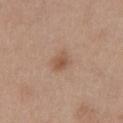{
  "biopsy_status": "not biopsied; imaged during a skin examination",
  "image": {
    "source": "total-body photography crop",
    "field_of_view_mm": 15
  },
  "lesion_size": {
    "long_diameter_mm_approx": 2.5
  },
  "patient": {
    "sex": "female",
    "age_approx": 40
  },
  "automated_metrics": {
    "area_mm2_approx": 4.5,
    "eccentricity": 0.5,
    "color_variation_0_10": 3.0,
    "nevus_likeness_0_100": 65
  },
  "lighting": "white-light",
  "site": "chest"
}